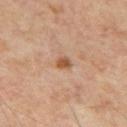follow-up=imaged on a skin check; not biopsied | tile lighting=cross-polarized illumination | lesion size=≈2 mm | location=the mid back | image=total-body-photography crop, ~15 mm field of view | patient=male, about 55 years old.